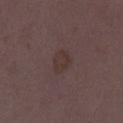This lesion was catalogued during total-body skin photography and was not selected for biopsy.